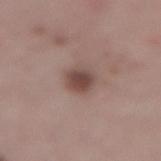Captured during whole-body skin photography for melanoma surveillance; the lesion was not biopsied. The tile uses white-light illumination. A female subject, aged 43–47. About 3 mm across. On the lower back. A close-up tile cropped from a whole-body skin photograph, about 15 mm across.